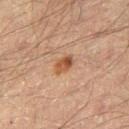The lesion was photographed on a routine skin check and not biopsied; there is no pathology result. Cropped from a whole-body photographic skin survey; the tile spans about 15 mm. The lesion is located on the right thigh. The lesion's longest dimension is about 3 mm. A male patient, approximately 60 years of age.